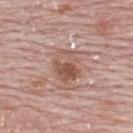– notes · total-body-photography surveillance lesion; no biopsy
– patient · female, aged 63 to 67
– image source · ~15 mm tile from a whole-body skin photo
– lesion diameter · about 4 mm
– lighting · white-light illumination
– location · the upper back